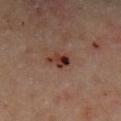Q: Is there a histopathology result?
A: catalogued during a skin exam; not biopsied
Q: Patient demographics?
A: male, in their mid-60s
Q: How was this image acquired?
A: total-body-photography crop, ~15 mm field of view
Q: Lesion size?
A: about 2.5 mm
Q: What did automated image analysis measure?
A: an automated nevus-likeness rating near 0 out of 100 and a lesion-detection confidence of about 100/100
Q: Lesion location?
A: the leg